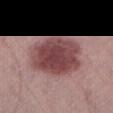{"biopsy_status": "not biopsied; imaged during a skin examination", "lighting": "white-light", "patient": {"sex": "male", "age_approx": 70}, "lesion_size": {"long_diameter_mm_approx": 6.5}, "site": "left thigh", "image": {"source": "total-body photography crop", "field_of_view_mm": 15}, "automated_metrics": {"eccentricity": 0.65, "shape_asymmetry": 0.1, "vs_skin_darker_L": 15.0, "vs_skin_contrast_norm": 11.0, "border_irregularity_0_10": 1.5, "color_variation_0_10": 4.5}}